Cropped from a whole-body photographic skin survey; the tile spans about 15 mm; the lesion is on the lower back; a female patient aged around 30: 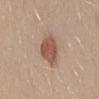Notes:
• tile lighting: white-light
• lesion size: about 3.5 mm
• automated lesion analysis: an eccentricity of roughly 0.45; a lesion color around L≈53 a*≈20 b*≈28 in CIELAB, roughly 12 lightness units darker than nearby skin, and a lesion-to-skin contrast of about 8 (normalized; higher = more distinct); a lesion-detection confidence of about 100/100
• diagnosis: a dysplastic (Clark) nevus